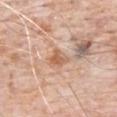biopsy status: imaged on a skin check; not biopsied
location: the chest
acquisition: 15 mm crop, total-body photography
patient: male, roughly 80 years of age